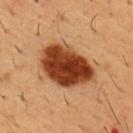size: ≈7 mm
patient: male, in their mid-50s
image source: 15 mm crop, total-body photography
site: the chest
tile lighting: cross-polarized